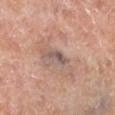Q: What is the anatomic site?
A: the left lower leg
Q: Who is the patient?
A: male, approximately 65 years of age
Q: How was the tile lit?
A: cross-polarized
Q: What kind of image is this?
A: ~15 mm tile from a whole-body skin photo